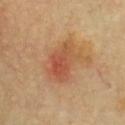Clinical impression:
This lesion was catalogued during total-body skin photography and was not selected for biopsy.
Context:
On the chest. Automated image analysis of the tile measured border irregularity of about 3.5 on a 0–10 scale and radial color variation of about 2. The software also gave a classifier nevus-likeness of about 95/100 and lesion-presence confidence of about 100/100. About 5.5 mm across. A 15 mm crop from a total-body photograph taken for skin-cancer surveillance. A male subject aged approximately 55.